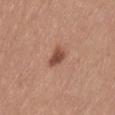Captured during whole-body skin photography for melanoma surveillance; the lesion was not biopsied. Cropped from a total-body skin-imaging series; the visible field is about 15 mm. The lesion is located on the left thigh. The patient is a female aged 33 to 37.